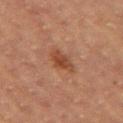The total-body-photography lesion software estimated a footprint of about 5 mm². It also reported a lesion color around L≈38 a*≈21 b*≈29 in CIELAB, about 8 CIELAB-L* units darker than the surrounding skin, and a normalized lesion–skin contrast near 7.5. This is a cross-polarized tile. The patient is a female aged around 70. On the right upper arm. A 15 mm crop from a total-body photograph taken for skin-cancer surveillance.